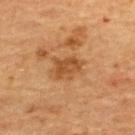Impression: This lesion was catalogued during total-body skin photography and was not selected for biopsy. Acquisition and patient details: A female patient, aged 68 to 72. Approximately 3.5 mm at its widest. On the back. A 15 mm close-up extracted from a 3D total-body photography capture.